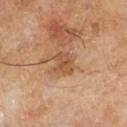Imaged during a routine full-body skin examination; the lesion was not biopsied and no histopathology is available. This is a cross-polarized tile. A male patient in their mid-60s. Cropped from a whole-body photographic skin survey; the tile spans about 15 mm. From the left lower leg. Automated image analysis of the tile measured a lesion area of about 5.5 mm² and an eccentricity of roughly 0.6. The software also gave a mean CIELAB color near L≈53 a*≈22 b*≈36 and a normalized lesion–skin contrast near 6.5. The software also gave a border-irregularity index near 4.5/10, a within-lesion color-variation index near 3/10, and radial color variation of about 1. The recorded lesion diameter is about 3 mm.